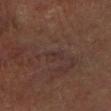Assessment: Recorded during total-body skin imaging; not selected for excision or biopsy. Image and clinical context: Automated image analysis of the tile measured about 4 CIELAB-L* units darker than the surrounding skin. The analysis additionally found a border-irregularity rating of about 5/10, a color-variation rating of about 0/10, and a peripheral color-asymmetry measure near 0. The recorded lesion diameter is about 2.5 mm. This is a cross-polarized tile. A close-up tile cropped from a whole-body skin photograph, about 15 mm across. From the right lower leg. A male subject, aged approximately 65.